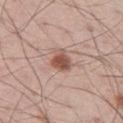Clinical summary: The lesion-visualizer software estimated an area of roughly 5.5 mm², a shape eccentricity near 0.5, and two-axis asymmetry of about 0.3. It also reported a classifier nevus-likeness of about 95/100 and a lesion-detection confidence of about 100/100. A male patient aged approximately 60. A lesion tile, about 15 mm wide, cut from a 3D total-body photograph. The lesion is on the left thigh. Imaged with white-light lighting.